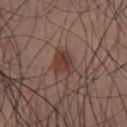Assessment: This lesion was catalogued during total-body skin photography and was not selected for biopsy. Clinical summary: From the chest. The subject is a male in their mid-30s. Cropped from a whole-body photographic skin survey; the tile spans about 15 mm. The recorded lesion diameter is about 3 mm. Imaged with white-light lighting.